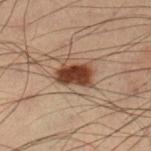Q: Was this lesion biopsied?
A: imaged on a skin check; not biopsied
Q: What is the lesion's diameter?
A: ≈4 mm
Q: Patient demographics?
A: male, roughly 55 years of age
Q: Lesion location?
A: the left lower leg
Q: Automated lesion metrics?
A: a footprint of about 9 mm², an outline eccentricity of about 0.8 (0 = round, 1 = elongated), and a symmetry-axis asymmetry near 0.2; roughly 15 lightness units darker than nearby skin; a within-lesion color-variation index near 3.5/10
Q: How was the tile lit?
A: cross-polarized illumination
Q: What is the imaging modality?
A: total-body-photography crop, ~15 mm field of view